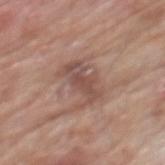Captured during whole-body skin photography for melanoma surveillance; the lesion was not biopsied.
The subject is a male aged 78 to 82.
Cropped from a whole-body photographic skin survey; the tile spans about 15 mm.
The lesion is on the mid back.